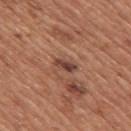notes — catalogued during a skin exam; not biopsied | location — the mid back | automated metrics — an area of roughly 3 mm², an outline eccentricity of about 0.85 (0 = round, 1 = elongated), and a symmetry-axis asymmetry near 0.35; a border-irregularity rating of about 3.5/10 and internal color variation of about 5 on a 0–10 scale; a nevus-likeness score of about 0/100 and lesion-presence confidence of about 100/100 | lighting — white-light illumination | image — ~15 mm crop, total-body skin-cancer survey | size — about 2.5 mm | patient — male, about 65 years old.